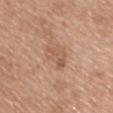Recorded during total-body skin imaging; not selected for excision or biopsy.
The total-body-photography lesion software estimated a lesion area of about 6 mm², an outline eccentricity of about 0.85 (0 = round, 1 = elongated), and a symmetry-axis asymmetry near 0.4. And it measured roughly 7 lightness units darker than nearby skin. And it measured border irregularity of about 4.5 on a 0–10 scale and a color-variation rating of about 3.5/10. It also reported a classifier nevus-likeness of about 0/100.
The lesion's longest dimension is about 4 mm.
A male patient, aged 38 to 42.
A 15 mm crop from a total-body photograph taken for skin-cancer surveillance.
This is a white-light tile.
Located on the upper back.